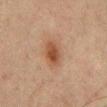Part of a total-body skin-imaging series; this lesion was reviewed on a skin check and was not flagged for biopsy. Longest diameter approximately 3.5 mm. A male patient, roughly 65 years of age. Captured under cross-polarized illumination. Automated image analysis of the tile measured an average lesion color of about L≈39 a*≈19 b*≈28 (CIELAB), about 10 CIELAB-L* units darker than the surrounding skin, and a normalized border contrast of about 8.5. The software also gave a nevus-likeness score of about 95/100. Cropped from a total-body skin-imaging series; the visible field is about 15 mm. Located on the mid back.